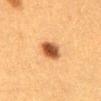Assessment: The lesion was tiled from a total-body skin photograph and was not biopsied. Acquisition and patient details: A female subject aged 38 to 42. Imaged with cross-polarized lighting. The lesion's longest dimension is about 3 mm. Automated tile analysis of the lesion measured an eccentricity of roughly 0.25. It also reported a border-irregularity index near 1/10, a within-lesion color-variation index near 5/10, and a peripheral color-asymmetry measure near 1.5. The analysis additionally found a lesion-detection confidence of about 100/100. A close-up tile cropped from a whole-body skin photograph, about 15 mm across. Located on the abdomen.